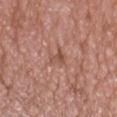The lesion was photographed on a routine skin check and not biopsied; there is no pathology result. The tile uses white-light illumination. The lesion's longest dimension is about 2.5 mm. Located on the head or neck. A male subject, aged around 50. A 15 mm close-up extracted from a 3D total-body photography capture. An algorithmic analysis of the crop reported a footprint of about 3.5 mm², an outline eccentricity of about 0.75 (0 = round, 1 = elongated), and a symmetry-axis asymmetry near 0.45.